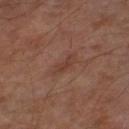<tbp_lesion>
<site>right lower leg</site>
<lighting>cross-polarized</lighting>
<image>
  <source>total-body photography crop</source>
  <field_of_view_mm>15</field_of_view_mm>
</image>
<patient>
  <sex>male</sex>
  <age_approx>65</age_approx>
</patient>
</tbp_lesion>